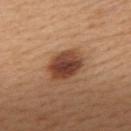Q: Was this lesion biopsied?
A: total-body-photography surveillance lesion; no biopsy
Q: How large is the lesion?
A: about 4.5 mm
Q: What are the patient's age and sex?
A: female, aged approximately 30
Q: Automated lesion metrics?
A: a lesion color around L≈42 a*≈23 b*≈30 in CIELAB, a lesion–skin lightness drop of about 15, and a normalized lesion–skin contrast near 11.5
Q: What is the anatomic site?
A: the upper back
Q: Illumination type?
A: white-light illumination
Q: What kind of image is this?
A: 15 mm crop, total-body photography